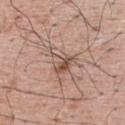The lesion was photographed on a routine skin check and not biopsied; there is no pathology result. A male subject aged 63–67. A roughly 15 mm field-of-view crop from a total-body skin photograph. The lesion is located on the upper back. The recorded lesion diameter is about 4 mm.